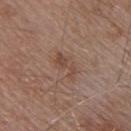workup — catalogued during a skin exam; not biopsied
patient — male, approximately 55 years of age
site — the upper back
tile lighting — white-light
image source — ~15 mm crop, total-body skin-cancer survey
automated metrics — a footprint of about 5 mm², a shape eccentricity near 0.85, and two-axis asymmetry of about 0.45; a lesion color around L≈46 a*≈18 b*≈26 in CIELAB, about 7 CIELAB-L* units darker than the surrounding skin, and a normalized border contrast of about 5.5; a classifier nevus-likeness of about 0/100 and lesion-presence confidence of about 100/100